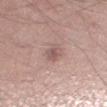Background: Automated tile analysis of the lesion measured a lesion area of about 4.5 mm² and a shape eccentricity near 0.75. And it measured internal color variation of about 2 on a 0–10 scale and peripheral color asymmetry of about 0.5. On the left lower leg. Captured under white-light illumination. A male subject aged 53 to 57. A 15 mm crop from a total-body photograph taken for skin-cancer surveillance. Approximately 3 mm at its widest.